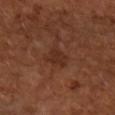Notes:
- follow-up: catalogued during a skin exam; not biopsied
- TBP lesion metrics: border irregularity of about 3.5 on a 0–10 scale, a color-variation rating of about 1.5/10, and radial color variation of about 0.5; a nevus-likeness score of about 0/100 and a detector confidence of about 100 out of 100 that the crop contains a lesion
- patient: female, approximately 65 years of age
- image source: 15 mm crop, total-body photography
- location: the right forearm